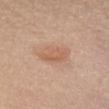The lesion was tiled from a total-body skin photograph and was not biopsied. The lesion is on the chest. Longest diameter approximately 4 mm. The patient is a female in their mid- to late 50s. A 15 mm close-up extracted from a 3D total-body photography capture. Automated tile analysis of the lesion measured a footprint of about 8 mm² and a symmetry-axis asymmetry near 0.2. The software also gave a lesion–skin lightness drop of about 8.